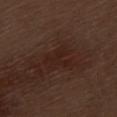The lesion was photographed on a routine skin check and not biopsied; there is no pathology result.
The subject is a male about 70 years old.
Imaged with white-light lighting.
Located on the left thigh.
Longest diameter approximately 3.5 mm.
A 15 mm close-up tile from a total-body photography series done for melanoma screening.
The lesion-visualizer software estimated roughly 4 lightness units darker than nearby skin and a lesion-to-skin contrast of about 5.5 (normalized; higher = more distinct). The software also gave an automated nevus-likeness rating near 0 out of 100 and a detector confidence of about 100 out of 100 that the crop contains a lesion.